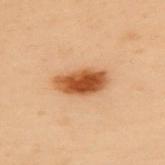workup = imaged on a skin check; not biopsied
anatomic site = the upper back
image source = ~15 mm tile from a whole-body skin photo
patient = female, about 60 years old